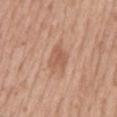This lesion was catalogued during total-body skin photography and was not selected for biopsy. The patient is a male aged 53–57. From the back. A 15 mm crop from a total-body photograph taken for skin-cancer surveillance. The lesion-visualizer software estimated an area of roughly 4.5 mm², an outline eccentricity of about 0.6 (0 = round, 1 = elongated), and two-axis asymmetry of about 0.5. And it measured border irregularity of about 5 on a 0–10 scale and radial color variation of about 0.5. The software also gave an automated nevus-likeness rating near 30 out of 100 and lesion-presence confidence of about 100/100. This is a white-light tile.